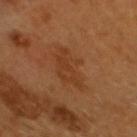Recorded during total-body skin imaging; not selected for excision or biopsy. A 15 mm crop from a total-body photograph taken for skin-cancer surveillance. On the head or neck. Imaged with cross-polarized lighting. A male patient in their 60s.